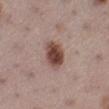{
  "biopsy_status": "not biopsied; imaged during a skin examination",
  "image": {
    "source": "total-body photography crop",
    "field_of_view_mm": 15
  },
  "automated_metrics": {
    "area_mm2_approx": 8.5,
    "eccentricity": 0.6,
    "border_irregularity_0_10": 1.5,
    "color_variation_0_10": 5.0,
    "peripheral_color_asymmetry": 1.5,
    "lesion_detection_confidence_0_100": 100
  },
  "patient": {
    "sex": "female",
    "age_approx": 55
  },
  "lesion_size": {
    "long_diameter_mm_approx": 3.5
  },
  "site": "left lower leg"
}